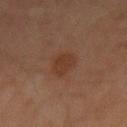follow-up: catalogued during a skin exam; not biopsied.